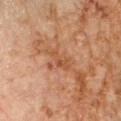Clinical impression: This lesion was catalogued during total-body skin photography and was not selected for biopsy. Context: This is a cross-polarized tile. A roughly 15 mm field-of-view crop from a total-body skin photograph. Automated tile analysis of the lesion measured an outline eccentricity of about 0.85 (0 = round, 1 = elongated) and a symmetry-axis asymmetry near 0.55. The software also gave a normalized border contrast of about 5. The software also gave a border-irregularity index near 6/10, a color-variation rating of about 0.5/10, and a peripheral color-asymmetry measure near 0. Longest diameter approximately 3 mm. From the right forearm. A male subject, aged around 60.